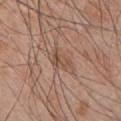| field | value |
|---|---|
| biopsy status | catalogued during a skin exam; not biopsied |